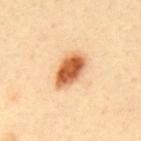| feature | finding |
|---|---|
| workup | catalogued during a skin exam; not biopsied |